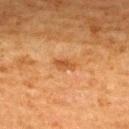No biopsy was performed on this lesion — it was imaged during a full skin examination and was not determined to be concerning.
A 15 mm close-up tile from a total-body photography series done for melanoma screening.
A female patient aged 48–52.
From the upper back.
Approximately 2.5 mm at its widest.
This is a cross-polarized tile.